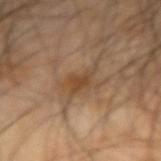No biopsy was performed on this lesion — it was imaged during a full skin examination and was not determined to be concerning.
A lesion tile, about 15 mm wide, cut from a 3D total-body photograph.
Automated image analysis of the tile measured roughly 8 lightness units darker than nearby skin and a normalized lesion–skin contrast near 6.5. The software also gave a nevus-likeness score of about 65/100 and a detector confidence of about 100 out of 100 that the crop contains a lesion.
From the right forearm.
A male subject, roughly 65 years of age.